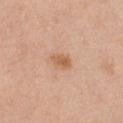Clinical impression:
The lesion was tiled from a total-body skin photograph and was not biopsied.
Clinical summary:
The lesion is located on the front of the torso. A female patient, roughly 40 years of age. A region of skin cropped from a whole-body photographic capture, roughly 15 mm wide. About 2.5 mm across.